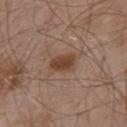Case summary:
* biopsy status — imaged on a skin check; not biopsied
* TBP lesion metrics — a footprint of about 5 mm², an outline eccentricity of about 0.75 (0 = round, 1 = elongated), and a symmetry-axis asymmetry near 0.25; a lesion color around L≈42 a*≈20 b*≈28 in CIELAB and roughly 11 lightness units darker than nearby skin; a classifier nevus-likeness of about 95/100 and a lesion-detection confidence of about 100/100
* location — the upper back
* lighting — white-light illumination
* image source — ~15 mm crop, total-body skin-cancer survey
* patient — male, approximately 55 years of age